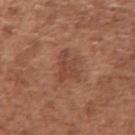Captured during whole-body skin photography for melanoma surveillance; the lesion was not biopsied. A female subject, roughly 50 years of age. The tile uses white-light illumination. From the arm. Cropped from a whole-body photographic skin survey; the tile spans about 15 mm.